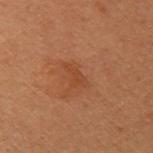Impression: The lesion was tiled from a total-body skin photograph and was not biopsied.